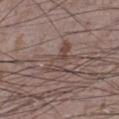Imaged during a routine full-body skin examination; the lesion was not biopsied and no histopathology is available. The lesion's longest dimension is about 5 mm. The subject is a male roughly 75 years of age. A 15 mm close-up extracted from a 3D total-body photography capture. Imaged with white-light lighting. The lesion is located on the left lower leg.